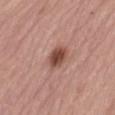Impression:
The lesion was tiled from a total-body skin photograph and was not biopsied.
Background:
Located on the right thigh. A male patient, aged approximately 75. Cropped from a total-body skin-imaging series; the visible field is about 15 mm. Approximately 3.5 mm at its widest.